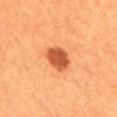The lesion was tiled from a total-body skin photograph and was not biopsied. The lesion is on the right thigh. This is a cross-polarized tile. A 15 mm close-up tile from a total-body photography series done for melanoma screening. A male patient aged approximately 60. Measured at roughly 3.5 mm in maximum diameter.